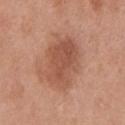Q: Was a biopsy performed?
A: catalogued during a skin exam; not biopsied
Q: What are the patient's age and sex?
A: male, in their 70s
Q: How was this image acquired?
A: 15 mm crop, total-body photography
Q: What is the anatomic site?
A: the front of the torso
Q: What is the lesion's diameter?
A: about 6.5 mm